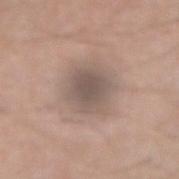  biopsy_status: not biopsied; imaged during a skin examination
  automated_metrics:
    area_mm2_approx: 15.0
    shape_asymmetry: 0.15
    vs_skin_darker_L: 10.0
    border_irregularity_0_10: 2.0
    color_variation_0_10: 2.5
    peripheral_color_asymmetry: 0.5
  image:
    source: total-body photography crop
    field_of_view_mm: 15
  lesion_size:
    long_diameter_mm_approx: 5.0
  lighting: white-light
  patient:
    sex: male
    age_approx: 65
  site: right forearm